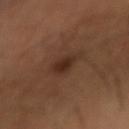Captured during whole-body skin photography for melanoma surveillance; the lesion was not biopsied.
The patient is a male aged around 50.
This image is a 15 mm lesion crop taken from a total-body photograph.
The total-body-photography lesion software estimated an average lesion color of about L≈28 a*≈18 b*≈25 (CIELAB), roughly 8 lightness units darker than nearby skin, and a lesion-to-skin contrast of about 8 (normalized; higher = more distinct). It also reported a border-irregularity index near 2/10 and peripheral color asymmetry of about 0.5. And it measured a classifier nevus-likeness of about 90/100.
The tile uses cross-polarized illumination.
The lesion is located on the right forearm.
Longest diameter approximately 2.5 mm.